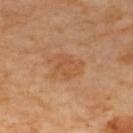Q: Is there a histopathology result?
A: catalogued during a skin exam; not biopsied
Q: Lesion size?
A: ~4.5 mm (longest diameter)
Q: What is the imaging modality?
A: total-body-photography crop, ~15 mm field of view
Q: What did automated image analysis measure?
A: a lesion color around L≈55 a*≈23 b*≈38 in CIELAB, roughly 7 lightness units darker than nearby skin, and a normalized lesion–skin contrast near 5; a border-irregularity index near 5/10 and a peripheral color-asymmetry measure near 0.5; an automated nevus-likeness rating near 0 out of 100
Q: Lesion location?
A: the upper back
Q: How was the tile lit?
A: cross-polarized